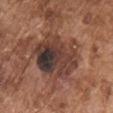| feature | finding |
|---|---|
| biopsy status | no biopsy performed (imaged during a skin exam) |
| subject | male, aged 73–77 |
| TBP lesion metrics | an area of roughly 26 mm², a shape eccentricity near 0.7, and two-axis asymmetry of about 0.2 |
| acquisition | ~15 mm crop, total-body skin-cancer survey |
| diameter | about 7 mm |
| tile lighting | white-light |
| anatomic site | the chest |